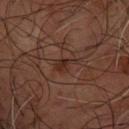biopsy_status: not biopsied; imaged during a skin examination
site: arm
image:
  source: total-body photography crop
  field_of_view_mm: 15
lighting: cross-polarized
patient:
  sex: male
  age_approx: 65
automated_metrics:
  border_irregularity_0_10: 5.5
  peripheral_color_asymmetry: 1.0